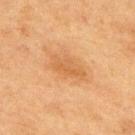Q: Is there a histopathology result?
A: total-body-photography surveillance lesion; no biopsy
Q: What is the anatomic site?
A: the upper back
Q: What are the patient's age and sex?
A: male, roughly 75 years of age
Q: How was the tile lit?
A: cross-polarized illumination
Q: Automated lesion metrics?
A: an area of roughly 7.5 mm², an eccentricity of roughly 0.85, and a symmetry-axis asymmetry near 0.2; a mean CIELAB color near L≈49 a*≈20 b*≈36 and about 7 CIELAB-L* units darker than the surrounding skin; a color-variation rating of about 2/10 and a peripheral color-asymmetry measure near 0.5; a nevus-likeness score of about 55/100 and a detector confidence of about 100 out of 100 that the crop contains a lesion
Q: How was this image acquired?
A: 15 mm crop, total-body photography
Q: What is the lesion's diameter?
A: ~4.5 mm (longest diameter)